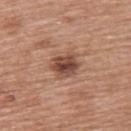workup: total-body-photography surveillance lesion; no biopsy | lesion diameter: ≈3.5 mm | site: the upper back | tile lighting: white-light illumination | imaging modality: total-body-photography crop, ~15 mm field of view | patient: female, aged around 60 | automated metrics: a footprint of about 8 mm², an eccentricity of roughly 0.55, and a symmetry-axis asymmetry near 0.2; a border-irregularity index near 2/10, a within-lesion color-variation index near 5.5/10, and radial color variation of about 1.5.